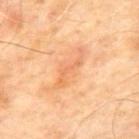| feature | finding |
|---|---|
| imaging modality | total-body-photography crop, ~15 mm field of view |
| patient | male, about 65 years old |
| site | the back |